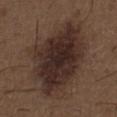biopsy status=total-body-photography surveillance lesion; no biopsy
image=~15 mm crop, total-body skin-cancer survey
location=the front of the torso
subject=male, aged approximately 50
lighting=white-light
size=~12.5 mm (longest diameter)